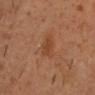Imaged during a routine full-body skin examination; the lesion was not biopsied and no histopathology is available.
Longest diameter approximately 4 mm.
On the head or neck.
The subject is a male aged 48–52.
Cropped from a whole-body photographic skin survey; the tile spans about 15 mm.